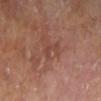  biopsy_status: not biopsied; imaged during a skin examination
  automated_metrics:
    nevus_likeness_0_100: 0
    lesion_detection_confidence_0_100: 100
  site: right lower leg
  patient:
    sex: female
    age_approx: 75
  image:
    source: total-body photography crop
    field_of_view_mm: 15
  lesion_size:
    long_diameter_mm_approx: 2.5
  lighting: cross-polarized